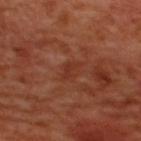Clinical impression:
The lesion was photographed on a routine skin check and not biopsied; there is no pathology result.
Context:
A region of skin cropped from a whole-body photographic capture, roughly 15 mm wide. Captured under cross-polarized illumination. The total-body-photography lesion software estimated a footprint of about 4 mm², an outline eccentricity of about 0.75 (0 = round, 1 = elongated), and two-axis asymmetry of about 0.3. The analysis additionally found border irregularity of about 3 on a 0–10 scale, a within-lesion color-variation index near 2/10, and peripheral color asymmetry of about 0.5. The analysis additionally found a lesion-detection confidence of about 100/100. The patient is a male aged 48 to 52. The recorded lesion diameter is about 3 mm. From the upper back.